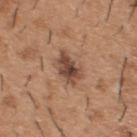| feature | finding |
|---|---|
| notes | total-body-photography surveillance lesion; no biopsy |
| image source | ~15 mm crop, total-body skin-cancer survey |
| lesion size | ≈4 mm |
| patient | male, aged approximately 40 |
| automated metrics | an outline eccentricity of about 0.75 (0 = round, 1 = elongated); roughly 12 lightness units darker than nearby skin and a lesion-to-skin contrast of about 9.5 (normalized; higher = more distinct) |
| lighting | white-light |
| body site | the back |